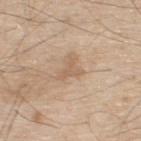Q: Is there a histopathology result?
A: total-body-photography surveillance lesion; no biopsy
Q: What is the imaging modality?
A: 15 mm crop, total-body photography
Q: Where on the body is the lesion?
A: the back
Q: What are the patient's age and sex?
A: male, aged 68–72